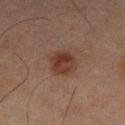The tile uses cross-polarized illumination. Cropped from a whole-body photographic skin survey; the tile spans about 15 mm. The patient is a male roughly 65 years of age. The lesion is located on the left thigh.